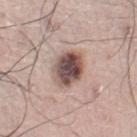Recorded during total-body skin imaging; not selected for excision or biopsy. A close-up tile cropped from a whole-body skin photograph, about 15 mm across. A male patient, approximately 70 years of age. The recorded lesion diameter is about 4 mm. This is a white-light tile. The lesion is on the left leg. An algorithmic analysis of the crop reported an area of roughly 12 mm², an outline eccentricity of about 0.55 (0 = round, 1 = elongated), and a shape-asymmetry score of about 0.15 (0 = symmetric). And it measured a border-irregularity rating of about 1.5/10, internal color variation of about 8.5 on a 0–10 scale, and peripheral color asymmetry of about 2.5. And it measured a detector confidence of about 100 out of 100 that the crop contains a lesion.